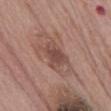Case summary:
– workup: catalogued during a skin exam; not biopsied
– imaging modality: ~15 mm tile from a whole-body skin photo
– body site: the abdomen
– patient: male, aged 68 to 72